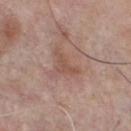Part of a total-body skin-imaging series; this lesion was reviewed on a skin check and was not flagged for biopsy.
Cropped from a total-body skin-imaging series; the visible field is about 15 mm.
The lesion is on the chest.
The patient is a male in their mid- to late 50s.
The recorded lesion diameter is about 3.5 mm.